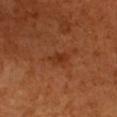Context: A 15 mm crop from a total-body photograph taken for skin-cancer surveillance. A female subject approximately 55 years of age. Imaged with cross-polarized lighting. Longest diameter approximately 3 mm. An algorithmic analysis of the crop reported a lesion area of about 3 mm², an eccentricity of roughly 0.9, and a symmetry-axis asymmetry near 0.25. The software also gave an automated nevus-likeness rating near 0 out of 100 and a lesion-detection confidence of about 100/100. From the chest.